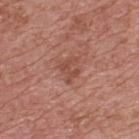Q: Was this lesion biopsied?
A: no biopsy performed (imaged during a skin exam)
Q: Illumination type?
A: white-light
Q: What is the lesion's diameter?
A: ≈3 mm
Q: Where on the body is the lesion?
A: the upper back
Q: What kind of image is this?
A: ~15 mm tile from a whole-body skin photo
Q: What are the patient's age and sex?
A: male, aged around 70